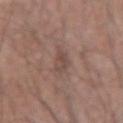| key | value |
|---|---|
| follow-up | total-body-photography surveillance lesion; no biopsy |
| site | the right forearm |
| subject | male, aged 48–52 |
| automated metrics | a border-irregularity rating of about 5.5/10 and a within-lesion color-variation index near 1.5/10 |
| acquisition | total-body-photography crop, ~15 mm field of view |
| illumination | white-light |